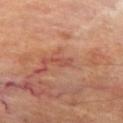notes: total-body-photography surveillance lesion; no biopsy | subject: male, aged 68 to 72 | illumination: cross-polarized illumination | automated metrics: a lesion–skin lightness drop of about 7 and a normalized lesion–skin contrast near 5.5 | lesion size: about 2.5 mm | acquisition: ~15 mm tile from a whole-body skin photo | site: the left thigh.